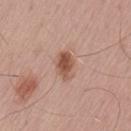Part of a total-body skin-imaging series; this lesion was reviewed on a skin check and was not flagged for biopsy. The subject is a male about 55 years old. Located on the lower back. Captured under white-light illumination. Cropped from a whole-body photographic skin survey; the tile spans about 15 mm. The recorded lesion diameter is about 3.5 mm. An algorithmic analysis of the crop reported a mean CIELAB color near L≈53 a*≈22 b*≈29, a lesion–skin lightness drop of about 12, and a normalized border contrast of about 8.5.